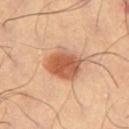Impression: Imaged during a routine full-body skin examination; the lesion was not biopsied and no histopathology is available. Clinical summary: From the left thigh. Measured at roughly 5 mm in maximum diameter. A 15 mm close-up extracted from a 3D total-body photography capture. Imaged with cross-polarized lighting. The lesion-visualizer software estimated an area of roughly 14 mm² and two-axis asymmetry of about 0.2. And it measured an average lesion color of about L≈60 a*≈25 b*≈37 (CIELAB), a lesion–skin lightness drop of about 15, and a normalized border contrast of about 9. It also reported a classifier nevus-likeness of about 100/100.